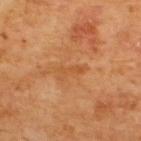Assessment:
The lesion was photographed on a routine skin check and not biopsied; there is no pathology result.
Context:
Imaged with cross-polarized lighting. Approximately 3 mm at its widest. A male patient roughly 65 years of age. Located on the back. Cropped from a whole-body photographic skin survey; the tile spans about 15 mm.